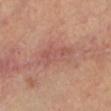biopsy status: catalogued during a skin exam; not biopsied | site: the left lower leg | lighting: cross-polarized | patient: female, about 25 years old | acquisition: ~15 mm tile from a whole-body skin photo.